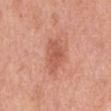The lesion was photographed on a routine skin check and not biopsied; there is no pathology result. Captured under white-light illumination. The recorded lesion diameter is about 5 mm. A lesion tile, about 15 mm wide, cut from a 3D total-body photograph. The lesion is located on the mid back. A male patient, aged 68–72.